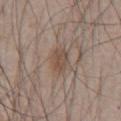Clinical impression:
This lesion was catalogued during total-body skin photography and was not selected for biopsy.
Clinical summary:
The lesion is located on the front of the torso. A 15 mm close-up extracted from a 3D total-body photography capture. A male patient, roughly 65 years of age.